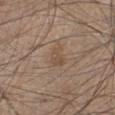Located on the left lower leg. A 15 mm close-up tile from a total-body photography series done for melanoma screening. A male subject aged approximately 45. The lesion-visualizer software estimated a lesion area of about 3.5 mm², a shape eccentricity near 0.75, and two-axis asymmetry of about 0.4. The software also gave internal color variation of about 1.5 on a 0–10 scale and radial color variation of about 0.5. The analysis additionally found a classifier nevus-likeness of about 25/100 and a detector confidence of about 100 out of 100 that the crop contains a lesion. The recorded lesion diameter is about 2.5 mm. The tile uses white-light illumination.